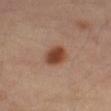{"biopsy_status": "not biopsied; imaged during a skin examination", "site": "left thigh", "patient": {"sex": "male", "age_approx": 55}, "automated_metrics": {"cielab_L": 42, "cielab_a": 22, "cielab_b": 29, "lesion_detection_confidence_0_100": 100}, "lesion_size": {"long_diameter_mm_approx": 3.0}, "image": {"source": "total-body photography crop", "field_of_view_mm": 15}}